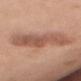biopsy status=imaged on a skin check; not biopsied
body site=the chest
diameter=~8.5 mm (longest diameter)
patient=female, roughly 50 years of age
illumination=white-light
acquisition=total-body-photography crop, ~15 mm field of view